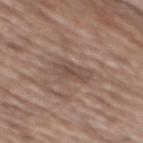follow-up — imaged on a skin check; not biopsied
diameter — ≈4 mm
automated metrics — a footprint of about 7.5 mm² and a symmetry-axis asymmetry near 0.35; internal color variation of about 3.5 on a 0–10 scale and radial color variation of about 1
patient — male, approximately 75 years of age
acquisition — ~15 mm crop, total-body skin-cancer survey
tile lighting — white-light illumination
location — the mid back